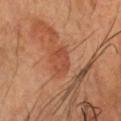Clinical impression: Captured during whole-body skin photography for melanoma surveillance; the lesion was not biopsied. Acquisition and patient details: The subject is a male aged around 60. Cropped from a whole-body photographic skin survey; the tile spans about 15 mm. Measured at roughly 3 mm in maximum diameter. Imaged with cross-polarized lighting. The lesion is located on the head or neck.